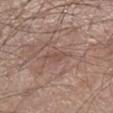follow-up = no biopsy performed (imaged during a skin exam); lesion size = ≈3.5 mm; site = the right lower leg; patient = male, approximately 60 years of age; imaging modality = ~15 mm tile from a whole-body skin photo; illumination = white-light illumination.